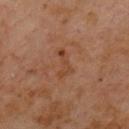Captured during whole-body skin photography for melanoma surveillance; the lesion was not biopsied. The subject is a male aged approximately 60. About 4 mm across. On the chest. The tile uses cross-polarized illumination. A 15 mm crop from a total-body photograph taken for skin-cancer surveillance. Automated image analysis of the tile measured a border-irregularity rating of about 7/10 and a within-lesion color-variation index near 0/10.